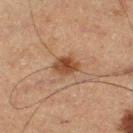Q: Was a biopsy performed?
A: imaged on a skin check; not biopsied
Q: What is the lesion's diameter?
A: about 3 mm
Q: What is the imaging modality?
A: ~15 mm tile from a whole-body skin photo
Q: What is the anatomic site?
A: the left thigh
Q: What lighting was used for the tile?
A: cross-polarized
Q: Patient demographics?
A: male, aged around 75